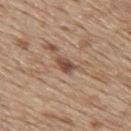biopsy status: no biopsy performed (imaged during a skin exam)
site: the upper back
imaging modality: total-body-photography crop, ~15 mm field of view
lighting: white-light
subject: male, aged approximately 70
lesion size: about 3 mm
automated lesion analysis: an average lesion color of about L≈49 a*≈18 b*≈28 (CIELAB), a lesion–skin lightness drop of about 12, and a normalized lesion–skin contrast near 8.5; border irregularity of about 3.5 on a 0–10 scale and a color-variation rating of about 3/10